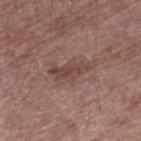follow-up=no biopsy performed (imaged during a skin exam)
site=the left thigh
image-analysis metrics=an area of roughly 9 mm² and two-axis asymmetry of about 0.45; an average lesion color of about L≈45 a*≈18 b*≈22 (CIELAB) and a lesion–skin lightness drop of about 8; border irregularity of about 5.5 on a 0–10 scale, internal color variation of about 3.5 on a 0–10 scale, and a peripheral color-asymmetry measure near 1.5
patient=male, approximately 70 years of age
acquisition=~15 mm tile from a whole-body skin photo
lighting=white-light illumination